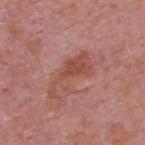Q: Was a biopsy performed?
A: catalogued during a skin exam; not biopsied
Q: What lighting was used for the tile?
A: white-light
Q: What is the imaging modality?
A: ~15 mm tile from a whole-body skin photo
Q: Lesion location?
A: the upper back
Q: Lesion size?
A: about 6 mm
Q: Patient demographics?
A: male, aged 73–77